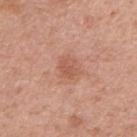workup = no biopsy performed (imaged during a skin exam)
TBP lesion metrics = two-axis asymmetry of about 0.15; an automated nevus-likeness rating near 5 out of 100
image = 15 mm crop, total-body photography
lighting = white-light
site = the right upper arm
subject = female, approximately 60 years of age